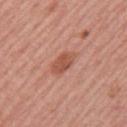Part of a total-body skin-imaging series; this lesion was reviewed on a skin check and was not flagged for biopsy.
The lesion is on the left upper arm.
A 15 mm close-up tile from a total-body photography series done for melanoma screening.
A female subject approximately 55 years of age.